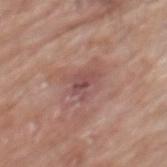biopsy status: no biopsy performed (imaged during a skin exam) | anatomic site: the mid back | acquisition: total-body-photography crop, ~15 mm field of view | size: ≈3 mm | subject: male, roughly 80 years of age.